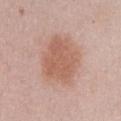Assessment: Captured during whole-body skin photography for melanoma surveillance; the lesion was not biopsied. Context: A close-up tile cropped from a whole-body skin photograph, about 15 mm across. A female subject about 50 years old. On the chest.